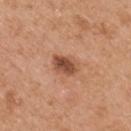workup = imaged on a skin check; not biopsied
anatomic site = the right upper arm
subject = male, aged 53 to 57
automated lesion analysis = a border-irregularity index near 2/10, a color-variation rating of about 4/10, and peripheral color asymmetry of about 1.5
diameter = ≈3.5 mm
acquisition = 15 mm crop, total-body photography
lighting = white-light